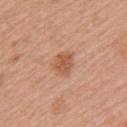| field | value |
|---|---|
| workup | catalogued during a skin exam; not biopsied |
| patient | female, aged around 55 |
| TBP lesion metrics | a lesion–skin lightness drop of about 10 and a normalized border contrast of about 7; a border-irregularity rating of about 1.5/10 and peripheral color asymmetry of about 1 |
| body site | the left upper arm |
| image source | 15 mm crop, total-body photography |
| lesion diameter | ≈3 mm |